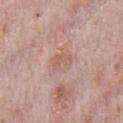biopsy status: catalogued during a skin exam; not biopsied | image: total-body-photography crop, ~15 mm field of view | subject: male, aged 68–72 | lesion size: ~2.5 mm (longest diameter) | lighting: white-light | site: the chest.